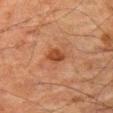| feature | finding |
|---|---|
| workup | total-body-photography surveillance lesion; no biopsy |
| tile lighting | cross-polarized |
| acquisition | ~15 mm crop, total-body skin-cancer survey |
| lesion size | ~2.5 mm (longest diameter) |
| patient | male, roughly 80 years of age |
| location | the left thigh |